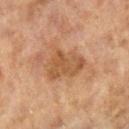This lesion was catalogued during total-body skin photography and was not selected for biopsy.
On the leg.
A region of skin cropped from a whole-body photographic capture, roughly 15 mm wide.
This is a cross-polarized tile.
About 5 mm across.
A female subject roughly 60 years of age.
The total-body-photography lesion software estimated a lesion color around L≈47 a*≈19 b*≈33 in CIELAB, a lesion–skin lightness drop of about 9, and a normalized border contrast of about 7. It also reported a border-irregularity rating of about 4/10, a within-lesion color-variation index near 3/10, and peripheral color asymmetry of about 1.